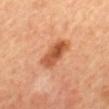The lesion was photographed on a routine skin check and not biopsied; there is no pathology result. About 5 mm across. A female subject, aged 58–62. Imaged with cross-polarized lighting. A lesion tile, about 15 mm wide, cut from a 3D total-body photograph. On the mid back.